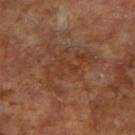A 15 mm close-up tile from a total-body photography series done for melanoma screening. On the arm. The patient is a male roughly 65 years of age. An algorithmic analysis of the crop reported a shape eccentricity near 0.8 and a shape-asymmetry score of about 0.55 (0 = symmetric). The analysis additionally found a mean CIELAB color near L≈36 a*≈20 b*≈29 and a normalized lesion–skin contrast near 5. And it measured a border-irregularity index near 9/10, internal color variation of about 3 on a 0–10 scale, and radial color variation of about 1. It also reported lesion-presence confidence of about 70/100. Longest diameter approximately 6 mm. Imaged with cross-polarized lighting.